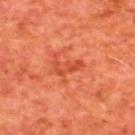biopsy status=total-body-photography surveillance lesion; no biopsy | TBP lesion metrics=an area of roughly 4 mm² and a symmetry-axis asymmetry near 0.5 | lighting=cross-polarized illumination | acquisition=total-body-photography crop, ~15 mm field of view | body site=the upper back | subject=male, approximately 65 years of age | lesion size=≈3 mm.